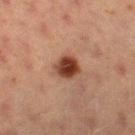| key | value |
|---|---|
| follow-up | imaged on a skin check; not biopsied |
| image source | total-body-photography crop, ~15 mm field of view |
| size | ~3 mm (longest diameter) |
| TBP lesion metrics | a within-lesion color-variation index near 5/10 and peripheral color asymmetry of about 2 |
| illumination | cross-polarized illumination |
| body site | the left thigh |
| subject | female, approximately 55 years of age |